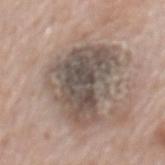– workup: total-body-photography surveillance lesion; no biopsy
– illumination: white-light
– patient: male, aged approximately 70
– imaging modality: 15 mm crop, total-body photography
– size: ≈7.5 mm
– location: the lower back